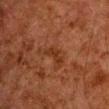The lesion was tiled from a total-body skin photograph and was not biopsied. The tile uses cross-polarized illumination. The lesion's longest dimension is about 3 mm. A 15 mm close-up extracted from a 3D total-body photography capture. A male patient approximately 60 years of age. On the chest.